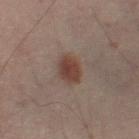This lesion was catalogued during total-body skin photography and was not selected for biopsy. From the right thigh. A male subject, in their 50s. A close-up tile cropped from a whole-body skin photograph, about 15 mm across. The tile uses cross-polarized illumination.